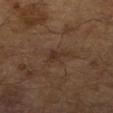Notes:
• biopsy status: total-body-photography surveillance lesion; no biopsy
• acquisition: total-body-photography crop, ~15 mm field of view
• subject: male, in their mid- to late 60s